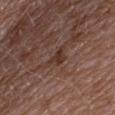Impression:
Imaged during a routine full-body skin examination; the lesion was not biopsied and no histopathology is available.
Context:
A female subject, approximately 60 years of age. The recorded lesion diameter is about 3.5 mm. Automated image analysis of the tile measured a shape eccentricity near 0.85 and two-axis asymmetry of about 0.5. It also reported border irregularity of about 5.5 on a 0–10 scale, internal color variation of about 2 on a 0–10 scale, and radial color variation of about 1. The analysis additionally found a classifier nevus-likeness of about 0/100. Located on the upper back. A 15 mm close-up extracted from a 3D total-body photography capture. Imaged with white-light lighting.